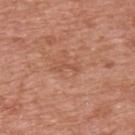Recorded during total-body skin imaging; not selected for excision or biopsy. Located on the upper back. A male patient, roughly 70 years of age. Imaged with white-light lighting. A roughly 15 mm field-of-view crop from a total-body skin photograph.